A male patient, roughly 50 years of age; imaged with white-light lighting; a region of skin cropped from a whole-body photographic capture, roughly 15 mm wide; Automated image analysis of the tile measured a lesion–skin lightness drop of about 15 and a normalized lesion–skin contrast near 10.5. The analysis additionally found a border-irregularity rating of about 3/10, internal color variation of about 8 on a 0–10 scale, and peripheral color asymmetry of about 2.5; from the left upper arm.
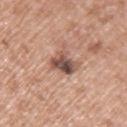Diagnosis: On biopsy, histopathology showed a dysplastic (Clark) nevus.